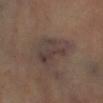Q: Is there a histopathology result?
A: total-body-photography surveillance lesion; no biopsy
Q: Automated lesion metrics?
A: internal color variation of about 4.5 on a 0–10 scale; an automated nevus-likeness rating near 0 out of 100 and a detector confidence of about 50 out of 100 that the crop contains a lesion
Q: Where on the body is the lesion?
A: the right lower leg
Q: How was this image acquired?
A: ~15 mm crop, total-body skin-cancer survey
Q: Who is the patient?
A: female, about 70 years old
Q: What is the lesion's diameter?
A: ≈6 mm
Q: Illumination type?
A: cross-polarized illumination